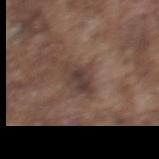notes: total-body-photography surveillance lesion; no biopsy
illumination: white-light
TBP lesion metrics: a mean CIELAB color near L≈37 a*≈14 b*≈20, a lesion–skin lightness drop of about 9, and a lesion-to-skin contrast of about 8.5 (normalized; higher = more distinct); a border-irregularity index near 3/10, internal color variation of about 2 on a 0–10 scale, and radial color variation of about 1; an automated nevus-likeness rating near 0 out of 100 and a detector confidence of about 80 out of 100 that the crop contains a lesion
anatomic site: the upper back
image source: ~15 mm crop, total-body skin-cancer survey
patient: male, roughly 75 years of age
lesion size: ≈4 mm